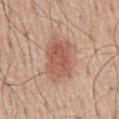Findings:
• workup — total-body-photography surveillance lesion; no biopsy
• image source — total-body-photography crop, ~15 mm field of view
• location — the mid back
• patient — male, aged approximately 60